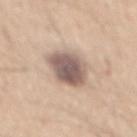biopsy status=total-body-photography surveillance lesion; no biopsy
location=the mid back
subject=male, aged 43 to 47
imaging modality=15 mm crop, total-body photography